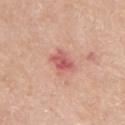Impression: The lesion was photographed on a routine skin check and not biopsied; there is no pathology result.